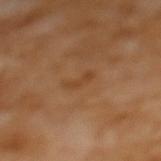Context:
The lesion is on the upper back. Automated tile analysis of the lesion measured peripheral color asymmetry of about 0. Cropped from a whole-body photographic skin survey; the tile spans about 15 mm. Approximately 3 mm at its widest. A female patient, aged 53–57. The tile uses cross-polarized illumination.